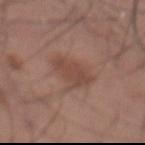| field | value |
|---|---|
| follow-up | catalogued during a skin exam; not biopsied |
| acquisition | ~15 mm tile from a whole-body skin photo |
| patient | male, aged approximately 55 |
| TBP lesion metrics | a lesion color around L≈46 a*≈20 b*≈25 in CIELAB, a lesion–skin lightness drop of about 8, and a normalized border contrast of about 6; a border-irregularity index near 4/10, a within-lesion color-variation index near 2/10, and radial color variation of about 0.5 |
| size | about 4 mm |
| anatomic site | the abdomen |
| tile lighting | white-light illumination |